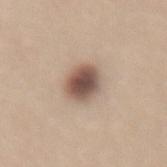Clinical impression: No biopsy was performed on this lesion — it was imaged during a full skin examination and was not determined to be concerning. Clinical summary: A close-up tile cropped from a whole-body skin photograph, about 15 mm across. Located on the lower back. A female patient, about 25 years old. This is a white-light tile.